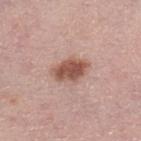{
  "automated_metrics": {
    "area_mm2_approx": 9.0,
    "eccentricity": 0.8
  },
  "lighting": "white-light",
  "patient": {
    "sex": "female",
    "age_approx": 65
  },
  "image": {
    "source": "total-body photography crop",
    "field_of_view_mm": 15
  },
  "lesion_size": {
    "long_diameter_mm_approx": 4.0
  },
  "site": "right lower leg"
}